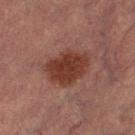biopsy status: total-body-photography surveillance lesion; no biopsy
site: the left lower leg
patient: female, approximately 70 years of age
acquisition: total-body-photography crop, ~15 mm field of view